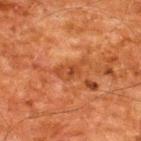This lesion was catalogued during total-body skin photography and was not selected for biopsy. This is a cross-polarized tile. The lesion-visualizer software estimated a lesion area of about 5.5 mm². And it measured an automated nevus-likeness rating near 0 out of 100. About 4 mm across. The patient is a male aged 58 to 62. From the back. This image is a 15 mm lesion crop taken from a total-body photograph.